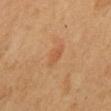| feature | finding |
|---|---|
| follow-up | no biopsy performed (imaged during a skin exam) |
| imaging modality | ~15 mm tile from a whole-body skin photo |
| TBP lesion metrics | an area of roughly 4 mm², an eccentricity of roughly 0.85, and a symmetry-axis asymmetry near 0.25; peripheral color asymmetry of about 0.5; a nevus-likeness score of about 10/100 and a detector confidence of about 100 out of 100 that the crop contains a lesion |
| patient | female, aged approximately 40 |
| body site | the mid back |
| lesion size | ≈3 mm |